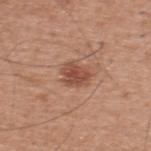Impression:
No biopsy was performed on this lesion — it was imaged during a full skin examination and was not determined to be concerning.
Clinical summary:
The total-body-photography lesion software estimated a mean CIELAB color near L≈49 a*≈23 b*≈29, roughly 11 lightness units darker than nearby skin, and a lesion-to-skin contrast of about 8 (normalized; higher = more distinct). The software also gave lesion-presence confidence of about 100/100. Cropped from a total-body skin-imaging series; the visible field is about 15 mm. The tile uses white-light illumination. Longest diameter approximately 3 mm. The lesion is on the upper back. The patient is a male aged 58–62.